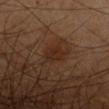Part of a total-body skin-imaging series; this lesion was reviewed on a skin check and was not flagged for biopsy. A male patient, aged 48–52. The lesion's longest dimension is about 3.5 mm. A lesion tile, about 15 mm wide, cut from a 3D total-body photograph. The total-body-photography lesion software estimated a lesion area of about 5.5 mm², an eccentricity of roughly 0.75, and a symmetry-axis asymmetry near 0.2. It also reported a classifier nevus-likeness of about 30/100 and a detector confidence of about 100 out of 100 that the crop contains a lesion. The tile uses cross-polarized illumination. On the left arm.